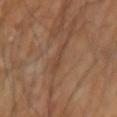Recorded during total-body skin imaging; not selected for excision or biopsy. Imaged with cross-polarized lighting. On the right forearm. Automated image analysis of the tile measured a footprint of about 3.5 mm², a shape eccentricity near 0.95, and a shape-asymmetry score of about 0.35 (0 = symmetric). The analysis additionally found a classifier nevus-likeness of about 0/100. Cropped from a whole-body photographic skin survey; the tile spans about 15 mm.